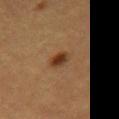Imaged during a routine full-body skin examination; the lesion was not biopsied and no histopathology is available. Located on the front of the torso. Automated image analysis of the tile measured a lesion color around L≈34 a*≈19 b*≈31 in CIELAB and a lesion-to-skin contrast of about 9.5 (normalized; higher = more distinct). The software also gave a border-irregularity rating of about 2/10 and radial color variation of about 1.5. A 15 mm crop from a total-body photograph taken for skin-cancer surveillance. Longest diameter approximately 2.5 mm. The subject is a male in their 60s.